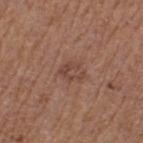This lesion was catalogued during total-body skin photography and was not selected for biopsy. The subject is a female in their 40s. On the leg. Measured at roughly 3 mm in maximum diameter. This image is a 15 mm lesion crop taken from a total-body photograph. This is a white-light tile.